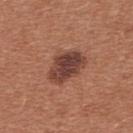Q: Was this lesion biopsied?
A: imaged on a skin check; not biopsied
Q: What lighting was used for the tile?
A: white-light illumination
Q: How was this image acquired?
A: ~15 mm tile from a whole-body skin photo
Q: How large is the lesion?
A: ≈5 mm
Q: Automated lesion metrics?
A: a mean CIELAB color near L≈42 a*≈23 b*≈25, about 13 CIELAB-L* units darker than the surrounding skin, and a normalized border contrast of about 10.5; an automated nevus-likeness rating near 65 out of 100 and a detector confidence of about 100 out of 100 that the crop contains a lesion
Q: Patient demographics?
A: female, aged approximately 35
Q: Where on the body is the lesion?
A: the chest